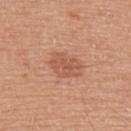Imaged during a routine full-body skin examination; the lesion was not biopsied and no histopathology is available.
The lesion is on the upper back.
The subject is a male about 55 years old.
The tile uses white-light illumination.
A 15 mm close-up extracted from a 3D total-body photography capture.